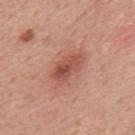This lesion was catalogued during total-body skin photography and was not selected for biopsy.
Imaged with white-light lighting.
Located on the mid back.
A male subject about 50 years old.
The lesion's longest dimension is about 4.5 mm.
A roughly 15 mm field-of-view crop from a total-body skin photograph.